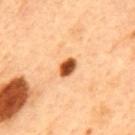<tbp_lesion>
  <biopsy_status>not biopsied; imaged during a skin examination</biopsy_status>
  <site>back</site>
  <patient>
    <sex>male</sex>
    <age_approx>50</age_approx>
  </patient>
  <lesion_size>
    <long_diameter_mm_approx>2.5</long_diameter_mm_approx>
  </lesion_size>
  <image>
    <source>total-body photography crop</source>
    <field_of_view_mm>15</field_of_view_mm>
  </image>
  <lighting>cross-polarized</lighting>
</tbp_lesion>